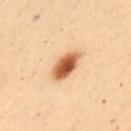Assessment:
The lesion was tiled from a total-body skin photograph and was not biopsied.
Background:
Captured under cross-polarized illumination. The subject is a female aged 48 to 52. The lesion is located on the front of the torso. A 15 mm close-up extracted from a 3D total-body photography capture.